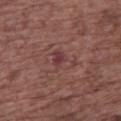workup=catalogued during a skin exam; not biopsied
subject=female, roughly 75 years of age
lighting=white-light illumination
body site=the upper back
acquisition=15 mm crop, total-body photography